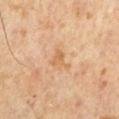Captured during whole-body skin photography for melanoma surveillance; the lesion was not biopsied. About 3 mm across. The subject is a male roughly 60 years of age. On the chest. This image is a 15 mm lesion crop taken from a total-body photograph. An algorithmic analysis of the crop reported an eccentricity of roughly 0.55 and a shape-asymmetry score of about 0.55 (0 = symmetric). The analysis additionally found a lesion color around L≈62 a*≈20 b*≈39 in CIELAB and a normalized lesion–skin contrast near 6. It also reported a border-irregularity rating of about 5/10 and radial color variation of about 0.5. It also reported a nevus-likeness score of about 0/100.